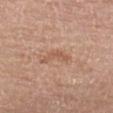Assessment:
The lesion was photographed on a routine skin check and not biopsied; there is no pathology result.
Context:
Imaged with white-light lighting. A female subject, roughly 85 years of age or older. The lesion-visualizer software estimated a border-irregularity rating of about 7/10 and a within-lesion color-variation index near 0/10. The analysis additionally found a classifier nevus-likeness of about 0/100 and a detector confidence of about 100 out of 100 that the crop contains a lesion. A lesion tile, about 15 mm wide, cut from a 3D total-body photograph. About 3.5 mm across. The lesion is on the back.